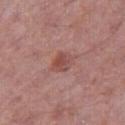The lesion was photographed on a routine skin check and not biopsied; there is no pathology result. The subject is a male approximately 70 years of age. Measured at roughly 2.5 mm in maximum diameter. Cropped from a total-body skin-imaging series; the visible field is about 15 mm. This is a white-light tile. On the right thigh. An algorithmic analysis of the crop reported a shape eccentricity near 0.65 and a symmetry-axis asymmetry near 0.2. The software also gave a lesion color around L≈49 a*≈24 b*≈24 in CIELAB. The analysis additionally found border irregularity of about 2 on a 0–10 scale and a within-lesion color-variation index near 2.5/10. And it measured a nevus-likeness score of about 45/100 and a detector confidence of about 100 out of 100 that the crop contains a lesion.